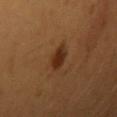<tbp_lesion>
<lighting>cross-polarized</lighting>
<lesion_size>
  <long_diameter_mm_approx>3.0</long_diameter_mm_approx>
</lesion_size>
<image>
  <source>total-body photography crop</source>
  <field_of_view_mm>15</field_of_view_mm>
</image>
<site>right upper arm</site>
<patient>
  <sex>female</sex>
  <age_approx>25</age_approx>
</patient>
</tbp_lesion>